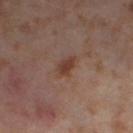<case>
<site>right thigh</site>
<image>
  <source>total-body photography crop</source>
  <field_of_view_mm>15</field_of_view_mm>
</image>
<patient>
  <sex>female</sex>
  <age_approx>55</age_approx>
</patient>
<lesion_size>
  <long_diameter_mm_approx>3.0</long_diameter_mm_approx>
</lesion_size>
<automated_metrics>
  <area_mm2_approx>3.5</area_mm2_approx>
  <eccentricity>0.85</eccentricity>
  <shape_asymmetry>0.3</shape_asymmetry>
  <cielab_L>39</cielab_L>
  <cielab_a>21</cielab_a>
  <cielab_b>27</cielab_b>
  <vs_skin_darker_L>9.0</vs_skin_darker_L>
  <vs_skin_contrast_norm>8.0</vs_skin_contrast_norm>
  <color_variation_0_10>2.5</color_variation_0_10>
  <peripheral_color_asymmetry>1.0</peripheral_color_asymmetry>
</automated_metrics>
</case>